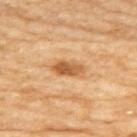Notes:
– notes · no biopsy performed (imaged during a skin exam)
– tile lighting · cross-polarized
– imaging modality · 15 mm crop, total-body photography
– subject · female, aged approximately 60
– body site · the upper back
– diameter · ≈3.5 mm